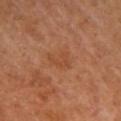The lesion was tiled from a total-body skin photograph and was not biopsied.